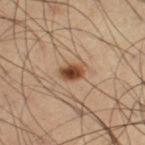Clinical summary:
A region of skin cropped from a whole-body photographic capture, roughly 15 mm wide. On the left thigh. This is a cross-polarized tile. The subject is a male approximately 65 years of age. The total-body-photography lesion software estimated a footprint of about 5 mm² and an eccentricity of roughly 0.75. The software also gave a nevus-likeness score of about 100/100 and lesion-presence confidence of about 100/100.